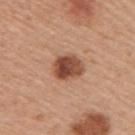This lesion was catalogued during total-body skin photography and was not selected for biopsy. The patient is a male aged around 55. The lesion is located on the back. A 15 mm close-up extracted from a 3D total-body photography capture. Automated tile analysis of the lesion measured an area of roughly 9.5 mm², a shape eccentricity near 0.6, and a shape-asymmetry score of about 0.1 (0 = symmetric). And it measured a lesion–skin lightness drop of about 15 and a normalized lesion–skin contrast near 10.5. And it measured border irregularity of about 1 on a 0–10 scale, internal color variation of about 6 on a 0–10 scale, and peripheral color asymmetry of about 2. The analysis additionally found a classifier nevus-likeness of about 80/100 and a lesion-detection confidence of about 100/100. Approximately 4 mm at its widest.